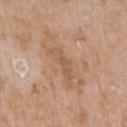Q: Was this lesion biopsied?
A: no biopsy performed (imaged during a skin exam)
Q: How was this image acquired?
A: total-body-photography crop, ~15 mm field of view
Q: Who is the patient?
A: female, in their mid-80s or older
Q: Where on the body is the lesion?
A: the left upper arm
Q: What did automated image analysis measure?
A: an outline eccentricity of about 0.95 (0 = round, 1 = elongated) and two-axis asymmetry of about 0.45; a lesion color around L≈56 a*≈20 b*≈33 in CIELAB, about 7 CIELAB-L* units darker than the surrounding skin, and a lesion-to-skin contrast of about 5 (normalized; higher = more distinct); border irregularity of about 6.5 on a 0–10 scale, internal color variation of about 0 on a 0–10 scale, and peripheral color asymmetry of about 0; an automated nevus-likeness rating near 0 out of 100 and a detector confidence of about 100 out of 100 that the crop contains a lesion
Q: What is the lesion's diameter?
A: ≈4 mm